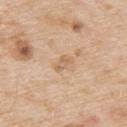No biopsy was performed on this lesion — it was imaged during a full skin examination and was not determined to be concerning.